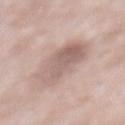The lesion was tiled from a total-body skin photograph and was not biopsied. A male subject approximately 55 years of age. About 5.5 mm across. Captured under white-light illumination. Located on the front of the torso. The total-body-photography lesion software estimated a mean CIELAB color near L≈61 a*≈17 b*≈22, a lesion–skin lightness drop of about 10, and a normalized border contrast of about 6. The analysis additionally found a border-irregularity index near 2/10, a within-lesion color-variation index near 5/10, and peripheral color asymmetry of about 2. The software also gave a lesion-detection confidence of about 100/100. A lesion tile, about 15 mm wide, cut from a 3D total-body photograph.